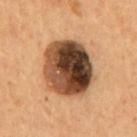notes: imaged on a skin check; not biopsied
patient: male, aged 53 to 57
tile lighting: cross-polarized illumination
site: the mid back
image-analysis metrics: an average lesion color of about L≈40 a*≈18 b*≈29 (CIELAB), roughly 23 lightness units darker than nearby skin, and a normalized border contrast of about 16.5; a border-irregularity index near 1/10, internal color variation of about 10 on a 0–10 scale, and peripheral color asymmetry of about 5.5
size: about 7 mm
imaging modality: 15 mm crop, total-body photography